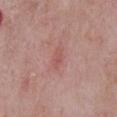Imaged during a routine full-body skin examination; the lesion was not biopsied and no histopathology is available. The recorded lesion diameter is about 3 mm. On the chest. A close-up tile cropped from a whole-body skin photograph, about 15 mm across. A male patient roughly 65 years of age. Imaged with white-light lighting.